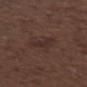This lesion was catalogued during total-body skin photography and was not selected for biopsy. About 2.5 mm across. A male patient about 50 years old. A 15 mm close-up tile from a total-body photography series done for melanoma screening. Located on the arm. Imaged with white-light lighting.